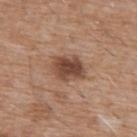<record>
  <biopsy_status>not biopsied; imaged during a skin examination</biopsy_status>
  <site>chest</site>
  <patient>
    <sex>male</sex>
    <age_approx>60</age_approx>
  </patient>
  <image>
    <source>total-body photography crop</source>
    <field_of_view_mm>15</field_of_view_mm>
  </image>
</record>